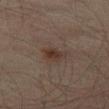The lesion was tiled from a total-body skin photograph and was not biopsied.